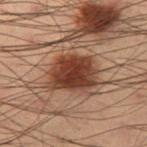No biopsy was performed on this lesion — it was imaged during a full skin examination and was not determined to be concerning. Imaged with cross-polarized lighting. A male subject about 55 years old. The lesion is on the left thigh. A 15 mm close-up tile from a total-body photography series done for melanoma screening.